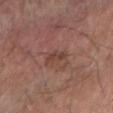lighting: white-light
automated_metrics:
  cielab_L: 42
  cielab_a: 21
  cielab_b: 24
  vs_skin_darker_L: 8.0
  vs_skin_contrast_norm: 6.5
  nevus_likeness_0_100: 35
  lesion_detection_confidence_0_100: 100
site: right forearm
image:
  source: total-body photography crop
  field_of_view_mm: 15
patient:
  sex: male
  age_approx: 70